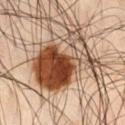Captured during whole-body skin photography for melanoma surveillance; the lesion was not biopsied.
A male patient, approximately 45 years of age.
The lesion is located on the right thigh.
A 15 mm close-up tile from a total-body photography series done for melanoma screening.